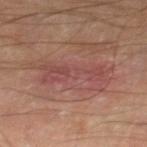biopsy status: total-body-photography surveillance lesion; no biopsy
image source: ~15 mm tile from a whole-body skin photo
size: ~8 mm (longest diameter)
subject: male, aged around 70
automated metrics: about 7 CIELAB-L* units darker than the surrounding skin and a lesion-to-skin contrast of about 6 (normalized; higher = more distinct); border irregularity of about 6.5 on a 0–10 scale and radial color variation of about 1
lighting: cross-polarized illumination
anatomic site: the right thigh